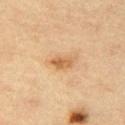- patient — female, aged around 65
- acquisition — ~15 mm tile from a whole-body skin photo
- body site — the right thigh
- lesion diameter — ~2.5 mm (longest diameter)
- tile lighting — cross-polarized